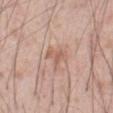Recorded during total-body skin imaging; not selected for excision or biopsy. About 3 mm across. An algorithmic analysis of the crop reported a footprint of about 5 mm², an eccentricity of roughly 0.6, and a symmetry-axis asymmetry near 0.55. The software also gave a lesion color around L≈60 a*≈20 b*≈28 in CIELAB, a lesion–skin lightness drop of about 8, and a normalized border contrast of about 5.5. And it measured a nevus-likeness score of about 0/100. The patient is a male in their mid- to late 50s. On the abdomen. Cropped from a whole-body photographic skin survey; the tile spans about 15 mm.